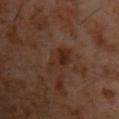follow-up = catalogued during a skin exam; not biopsied | tile lighting = cross-polarized | diameter = ~5 mm (longest diameter) | acquisition = total-body-photography crop, ~15 mm field of view | subject = male, aged 58 to 62 | location = the chest.